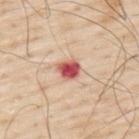{
  "image": {
    "source": "total-body photography crop",
    "field_of_view_mm": 15
  },
  "patient": {
    "sex": "male",
    "age_approx": 80
  },
  "automated_metrics": {
    "eccentricity": 0.55,
    "shape_asymmetry": 0.25,
    "nevus_likeness_0_100": 0,
    "lesion_detection_confidence_0_100": 100
  },
  "lighting": "white-light",
  "site": "upper back"
}